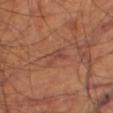Part of a total-body skin-imaging series; this lesion was reviewed on a skin check and was not flagged for biopsy.
The total-body-photography lesion software estimated a border-irregularity index near 5/10 and a within-lesion color-variation index near 2/10. And it measured a nevus-likeness score of about 0/100 and lesion-presence confidence of about 85/100.
From the leg.
Captured under cross-polarized illumination.
A male patient, aged approximately 70.
A close-up tile cropped from a whole-body skin photograph, about 15 mm across.
The lesion's longest dimension is about 3 mm.